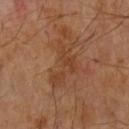Notes:
– workup: no biopsy performed (imaged during a skin exam)
– illumination: cross-polarized
– lesion diameter: ≈5.5 mm
– acquisition: 15 mm crop, total-body photography
– body site: the left forearm